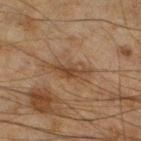follow-up = imaged on a skin check; not biopsied | image source = ~15 mm crop, total-body skin-cancer survey | location = the left lower leg | lighting = cross-polarized illumination | patient = male, aged 43–47.